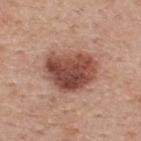Q: Was a biopsy performed?
A: imaged on a skin check; not biopsied
Q: How was the tile lit?
A: white-light
Q: Where on the body is the lesion?
A: the upper back
Q: Who is the patient?
A: male, aged approximately 60
Q: What did automated image analysis measure?
A: a footprint of about 22 mm², an eccentricity of roughly 0.65, and a shape-asymmetry score of about 0.2 (0 = symmetric); a classifier nevus-likeness of about 95/100 and a lesion-detection confidence of about 100/100
Q: What kind of image is this?
A: 15 mm crop, total-body photography
Q: What is the lesion's diameter?
A: about 6 mm